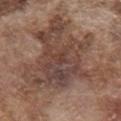Captured during whole-body skin photography for melanoma surveillance; the lesion was not biopsied. The patient is a male aged 73–77. Longest diameter approximately 12 mm. The lesion is located on the chest. Cropped from a total-body skin-imaging series; the visible field is about 15 mm. Automated image analysis of the tile measured a lesion area of about 65 mm² and a shape eccentricity near 0.5. The analysis additionally found border irregularity of about 9 on a 0–10 scale, a within-lesion color-variation index near 6.5/10, and a peripheral color-asymmetry measure near 2.